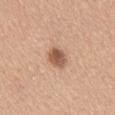<lesion>
<biopsy_status>not biopsied; imaged during a skin examination</biopsy_status>
<site>mid back</site>
<patient>
  <sex>female</sex>
  <age_approx>40</age_approx>
</patient>
<image>
  <source>total-body photography crop</source>
  <field_of_view_mm>15</field_of_view_mm>
</image>
</lesion>